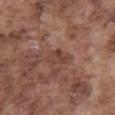Clinical impression:
This lesion was catalogued during total-body skin photography and was not selected for biopsy.